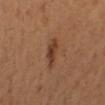Background: The subject is a female aged around 55. Captured under cross-polarized illumination. A roughly 15 mm field-of-view crop from a total-body skin photograph. From the left lower leg. Automated image analysis of the tile measured a lesion color around L≈40 a*≈21 b*≈31 in CIELAB and about 9 CIELAB-L* units darker than the surrounding skin. And it measured a classifier nevus-likeness of about 85/100 and lesion-presence confidence of about 100/100.